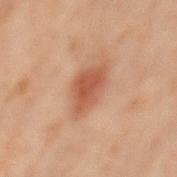Clinical impression: No biopsy was performed on this lesion — it was imaged during a full skin examination and was not determined to be concerning. Clinical summary: A roughly 15 mm field-of-view crop from a total-body skin photograph. The patient is a male aged approximately 60. On the lower back. Measured at roughly 5 mm in maximum diameter.